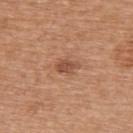Findings:
* biopsy status — no biopsy performed (imaged during a skin exam)
* image — 15 mm crop, total-body photography
* patient — female, in their mid-60s
* body site — the upper back
* size — about 3 mm
* tile lighting — white-light